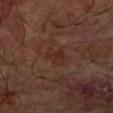No biopsy was performed on this lesion — it was imaged during a full skin examination and was not determined to be concerning.